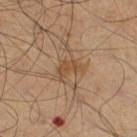This is a cross-polarized tile. Automated image analysis of the tile measured a border-irregularity index near 4.5/10, internal color variation of about 1 on a 0–10 scale, and a peripheral color-asymmetry measure near 0. Cropped from a total-body skin-imaging series; the visible field is about 15 mm. A male patient, aged 48–52. Approximately 3 mm at its widest. The lesion is on the left leg.